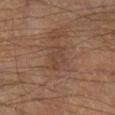Findings:
- biopsy status · no biopsy performed (imaged during a skin exam)
- image source · 15 mm crop, total-body photography
- diameter · about 3.5 mm
- subject · male, aged around 70
- anatomic site · the right lower leg
- image-analysis metrics · an outline eccentricity of about 0.85 (0 = round, 1 = elongated) and two-axis asymmetry of about 0.4; a border-irregularity index near 4.5/10 and a within-lesion color-variation index near 2/10; lesion-presence confidence of about 95/100
- illumination · cross-polarized